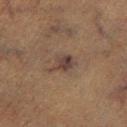follow-up = total-body-photography surveillance lesion; no biopsy | automated metrics = an eccentricity of roughly 0.75 and two-axis asymmetry of about 0.35; an average lesion color of about L≈32 a*≈13 b*≈19 (CIELAB) and a normalized lesion–skin contrast near 8; a border-irregularity rating of about 4/10 and a within-lesion color-variation index near 4/10 | patient = male, roughly 75 years of age | site = the left lower leg | acquisition = 15 mm crop, total-body photography | illumination = cross-polarized.